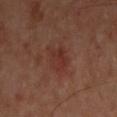The lesion was photographed on a routine skin check and not biopsied; there is no pathology result. A 15 mm close-up extracted from a 3D total-body photography capture. The total-body-photography lesion software estimated a mean CIELAB color near L≈32 a*≈23 b*≈24 and a normalized lesion–skin contrast near 5.5. It also reported internal color variation of about 3.5 on a 0–10 scale and peripheral color asymmetry of about 1. The software also gave a classifier nevus-likeness of about 75/100 and lesion-presence confidence of about 100/100. The tile uses cross-polarized illumination. The recorded lesion diameter is about 4 mm. From the front of the torso. The patient is a male aged approximately 50.